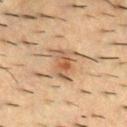workup: imaged on a skin check; not biopsied | illumination: cross-polarized illumination | lesion diameter: ~4.5 mm (longest diameter) | anatomic site: the upper back | patient: male, approximately 55 years of age | TBP lesion metrics: an area of roughly 12 mm² and a shape-asymmetry score of about 0.35 (0 = symmetric); a mean CIELAB color near L≈51 a*≈16 b*≈30 and a lesion–skin lightness drop of about 9; a within-lesion color-variation index near 7/10 and a peripheral color-asymmetry measure near 2; an automated nevus-likeness rating near 45 out of 100 and lesion-presence confidence of about 100/100 | image: 15 mm crop, total-body photography.